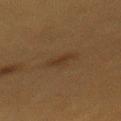Background: The lesion is on the back. Cropped from a total-body skin-imaging series; the visible field is about 15 mm. A female subject, about 40 years old.